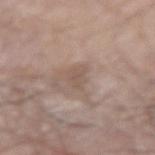{"biopsy_status": "not biopsied; imaged during a skin examination", "site": "right arm", "lesion_size": {"long_diameter_mm_approx": 2.5}, "lighting": "white-light", "image": {"source": "total-body photography crop", "field_of_view_mm": 15}, "patient": {"sex": "male", "age_approx": 80}}